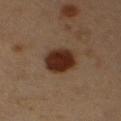TBP lesion metrics = a footprint of about 11 mm², an eccentricity of roughly 0.6, and two-axis asymmetry of about 0.15; border irregularity of about 1.5 on a 0–10 scale, a color-variation rating of about 3/10, and peripheral color asymmetry of about 1; a detector confidence of about 100 out of 100 that the crop contains a lesion | subject = male, about 55 years old | image source = total-body-photography crop, ~15 mm field of view | diameter = ≈4 mm | site = the right upper arm.